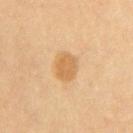<record>
  <biopsy_status>not biopsied; imaged during a skin examination</biopsy_status>
  <patient>
    <sex>male</sex>
    <age_approx>60</age_approx>
  </patient>
  <site>chest</site>
  <image>
    <source>total-body photography crop</source>
    <field_of_view_mm>15</field_of_view_mm>
  </image>
</record>